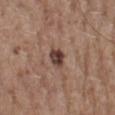Impression: Captured during whole-body skin photography for melanoma surveillance; the lesion was not biopsied. Context: Automated image analysis of the tile measured a mean CIELAB color near L≈40 a*≈18 b*≈22 and a normalized lesion–skin contrast near 11.5. The analysis additionally found a nevus-likeness score of about 55/100 and lesion-presence confidence of about 100/100. A male subject, aged approximately 75. From the mid back. Measured at roughly 3 mm in maximum diameter. Cropped from a whole-body photographic skin survey; the tile spans about 15 mm. The tile uses white-light illumination.